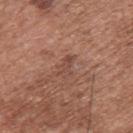This lesion was catalogued during total-body skin photography and was not selected for biopsy. Located on the upper back. About 2.5 mm across. Cropped from a total-body skin-imaging series; the visible field is about 15 mm. The subject is a male about 75 years old.